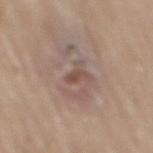Recorded during total-body skin imaging; not selected for excision or biopsy. A male subject, aged around 80. Captured under white-light illumination. Cropped from a total-body skin-imaging series; the visible field is about 15 mm. From the mid back. The recorded lesion diameter is about 3 mm.